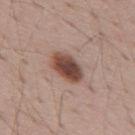Clinical impression: The lesion was tiled from a total-body skin photograph and was not biopsied. Background: Approximately 4.5 mm at its widest. A male subject aged 68 to 72. This is a white-light tile. The lesion is located on the mid back. This image is a 15 mm lesion crop taken from a total-body photograph.